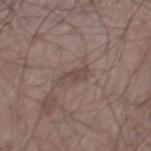{"biopsy_status": "not biopsied; imaged during a skin examination", "site": "chest", "patient": {"sex": "male", "age_approx": 60}, "image": {"source": "total-body photography crop", "field_of_view_mm": 15}}